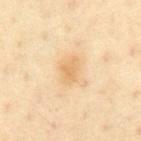  biopsy_status: not biopsied; imaged during a skin examination
  lesion_size:
    long_diameter_mm_approx: 3.0
  site: front of the torso
  patient:
    sex: male
    age_approx: 65
  image:
    source: total-body photography crop
    field_of_view_mm: 15
  lighting: cross-polarized
  automated_metrics:
    cielab_L: 70
    cielab_a: 16
    cielab_b: 41
    vs_skin_darker_L: 8.0
    nevus_likeness_0_100: 25
    lesion_detection_confidence_0_100: 100